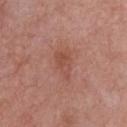This lesion was catalogued during total-body skin photography and was not selected for biopsy. A male subject, about 55 years old. Cropped from a whole-body photographic skin survey; the tile spans about 15 mm. On the chest.